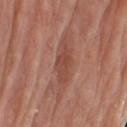workup: total-body-photography surveillance lesion; no biopsy
location: the right upper arm
image: 15 mm crop, total-body photography
subject: male, aged 78–82